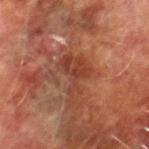Notes:
- notes: imaged on a skin check; not biopsied
- patient: male, in their mid- to late 70s
- TBP lesion metrics: an automated nevus-likeness rating near 0 out of 100 and a detector confidence of about 95 out of 100 that the crop contains a lesion
- body site: the leg
- acquisition: total-body-photography crop, ~15 mm field of view